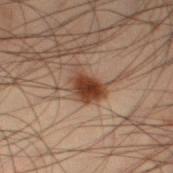| feature | finding |
|---|---|
| follow-up | catalogued during a skin exam; not biopsied |
| illumination | cross-polarized |
| site | the left thigh |
| subject | male, aged 53 to 57 |
| image | total-body-photography crop, ~15 mm field of view |
| diameter | ≈4 mm |